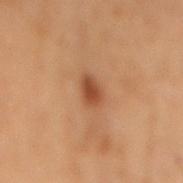biopsy status: no biopsy performed (imaged during a skin exam)
diameter: about 2.5 mm
site: the lower back
illumination: cross-polarized
image-analysis metrics: a footprint of about 4 mm², an eccentricity of roughly 0.75, and a shape-asymmetry score of about 0.2 (0 = symmetric); a nevus-likeness score of about 95/100 and a lesion-detection confidence of about 100/100
subject: male, aged around 70
image: ~15 mm crop, total-body skin-cancer survey